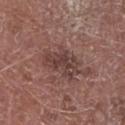Q: How was this image acquired?
A: total-body-photography crop, ~15 mm field of view
Q: What is the anatomic site?
A: the left lower leg
Q: How was the tile lit?
A: white-light illumination
Q: What did automated image analysis measure?
A: a border-irregularity rating of about 4.5/10; an automated nevus-likeness rating near 0 out of 100 and lesion-presence confidence of about 100/100
Q: Patient demographics?
A: male, in their mid- to late 70s
Q: What is the lesion's diameter?
A: about 5 mm